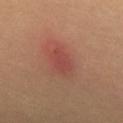{
  "biopsy_status": "not biopsied; imaged during a skin examination",
  "site": "upper back",
  "lighting": "cross-polarized",
  "lesion_size": {
    "long_diameter_mm_approx": 3.5
  },
  "patient": {
    "sex": "female",
    "age_approx": 60
  },
  "image": {
    "source": "total-body photography crop",
    "field_of_view_mm": 15
  }
}